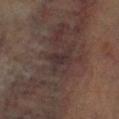This lesion was catalogued during total-body skin photography and was not selected for biopsy. This is a cross-polarized tile. A male subject approximately 70 years of age. A 15 mm close-up tile from a total-body photography series done for melanoma screening. Measured at roughly 3 mm in maximum diameter. Located on the left lower leg.